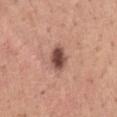Part of a total-body skin-imaging series; this lesion was reviewed on a skin check and was not flagged for biopsy. Approximately 3.5 mm at its widest. Cropped from a whole-body photographic skin survey; the tile spans about 15 mm. The tile uses white-light illumination. A male subject aged 43–47. Located on the chest. The lesion-visualizer software estimated a symmetry-axis asymmetry near 0.2. The software also gave an average lesion color of about L≈49 a*≈22 b*≈25 (CIELAB) and a normalized border contrast of about 11. The software also gave border irregularity of about 2 on a 0–10 scale, a color-variation rating of about 4/10, and radial color variation of about 1.5. And it measured a nevus-likeness score of about 90/100 and lesion-presence confidence of about 100/100.